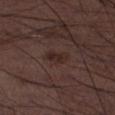No biopsy was performed on this lesion — it was imaged during a full skin examination and was not determined to be concerning. This is a white-light tile. Approximately 3 mm at its widest. A 15 mm crop from a total-body photograph taken for skin-cancer surveillance. A male patient, aged around 50. Located on the left lower leg.